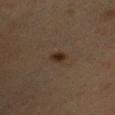  biopsy_status: not biopsied; imaged during a skin examination
  image:
    source: total-body photography crop
    field_of_view_mm: 15
  site: chest
  patient:
    sex: female
    age_approx: 40